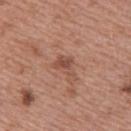Impression: No biopsy was performed on this lesion — it was imaged during a full skin examination and was not determined to be concerning. Image and clinical context: The tile uses white-light illumination. Longest diameter approximately 3 mm. From the upper back. A female subject, aged 38 to 42. A 15 mm crop from a total-body photograph taken for skin-cancer surveillance.